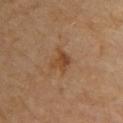The lesion was photographed on a routine skin check and not biopsied; there is no pathology result.
Longest diameter approximately 2.5 mm.
A 15 mm crop from a total-body photograph taken for skin-cancer surveillance.
The lesion is located on the upper back.
Captured under cross-polarized illumination.
An algorithmic analysis of the crop reported a mean CIELAB color near L≈43 a*≈20 b*≈34, a lesion–skin lightness drop of about 8, and a normalized border contrast of about 7. It also reported internal color variation of about 3 on a 0–10 scale and a peripheral color-asymmetry measure near 1.
A male subject, aged around 85 or older.